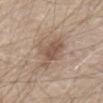<tbp_lesion>
  <biopsy_status>not biopsied; imaged during a skin examination</biopsy_status>
</tbp_lesion>